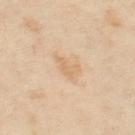{
  "biopsy_status": "not biopsied; imaged during a skin examination",
  "site": "left thigh",
  "automated_metrics": {
    "color_variation_0_10": 1.0,
    "peripheral_color_asymmetry": 0.5
  },
  "patient": {
    "sex": "female",
    "age_approx": 50
  },
  "lighting": "cross-polarized",
  "image": {
    "source": "total-body photography crop",
    "field_of_view_mm": 15
  },
  "lesion_size": {
    "long_diameter_mm_approx": 3.0
  }
}